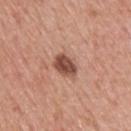Clinical impression:
Recorded during total-body skin imaging; not selected for excision or biopsy.
Context:
From the upper back. About 3 mm across. This is a white-light tile. A 15 mm close-up extracted from a 3D total-body photography capture. A male subject, aged 58 to 62.